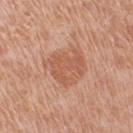Recorded during total-body skin imaging; not selected for excision or biopsy.
From the right upper arm.
This image is a 15 mm lesion crop taken from a total-body photograph.
A female patient in their mid- to late 50s.